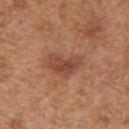Clinical impression:
No biopsy was performed on this lesion — it was imaged during a full skin examination and was not determined to be concerning.
Context:
A female subject, roughly 40 years of age. From the right upper arm. Imaged with white-light lighting. Longest diameter approximately 4 mm. A 15 mm crop from a total-body photograph taken for skin-cancer surveillance.